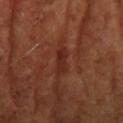The lesion was tiled from a total-body skin photograph and was not biopsied.
Longest diameter approximately 3.5 mm.
A close-up tile cropped from a whole-body skin photograph, about 15 mm across.
From the head or neck.
Automated tile analysis of the lesion measured an area of roughly 4 mm² and an outline eccentricity of about 0.95 (0 = round, 1 = elongated). And it measured a classifier nevus-likeness of about 0/100 and a lesion-detection confidence of about 95/100.